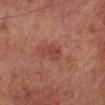Q: Was this lesion biopsied?
A: catalogued during a skin exam; not biopsied
Q: What are the patient's age and sex?
A: male, approximately 70 years of age
Q: How was the tile lit?
A: cross-polarized illumination
Q: What is the imaging modality?
A: ~15 mm tile from a whole-body skin photo
Q: Lesion size?
A: ~3 mm (longest diameter)
Q: What is the anatomic site?
A: the left lower leg